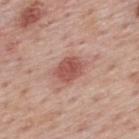anatomic site: the upper back | image source: 15 mm crop, total-body photography | subject: male, in their mid-70s.